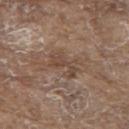Q: Is there a histopathology result?
A: no biopsy performed (imaged during a skin exam)
Q: Who is the patient?
A: male, roughly 80 years of age
Q: How was this image acquired?
A: 15 mm crop, total-body photography
Q: What is the lesion's diameter?
A: ~3.5 mm (longest diameter)
Q: Where on the body is the lesion?
A: the upper back